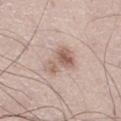An algorithmic analysis of the crop reported an area of roughly 9 mm², an eccentricity of roughly 0.85, and a shape-asymmetry score of about 0.5 (0 = symmetric).
A 15 mm crop from a total-body photograph taken for skin-cancer surveillance.
Captured under white-light illumination.
A male subject in their 50s.
Located on the right thigh.